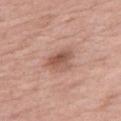| field | value |
|---|---|
| workup | imaged on a skin check; not biopsied |